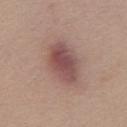body site: the chest
automated metrics: a lesion area of about 16 mm² and an outline eccentricity of about 0.8 (0 = round, 1 = elongated)
subject: female, aged approximately 60
image source: ~15 mm crop, total-body skin-cancer survey